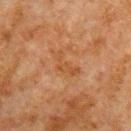The lesion was photographed on a routine skin check and not biopsied; there is no pathology result.
Located on the arm.
The subject is a male aged approximately 80.
A 15 mm close-up extracted from a 3D total-body photography capture.